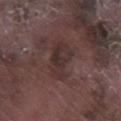The lesion was photographed on a routine skin check and not biopsied; there is no pathology result. A male patient roughly 75 years of age. A 15 mm close-up extracted from a 3D total-body photography capture. Imaged with white-light lighting. Automated tile analysis of the lesion measured a lesion area of about 10 mm², an eccentricity of roughly 0.85, and a symmetry-axis asymmetry near 0.3. And it measured an average lesion color of about L≈31 a*≈17 b*≈16 (CIELAB), a lesion–skin lightness drop of about 6, and a normalized border contrast of about 6.5. The recorded lesion diameter is about 5 mm. Located on the right lower leg.